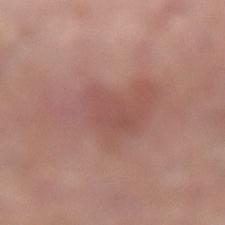The lesion was photographed on a routine skin check and not biopsied; there is no pathology result.
The subject is a male aged 63 to 67.
Longest diameter approximately 10 mm.
The tile uses white-light illumination.
Cropped from a whole-body photographic skin survey; the tile spans about 15 mm.
From the left lower leg.